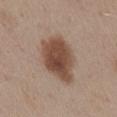This lesion was catalogued during total-body skin photography and was not selected for biopsy. Automated tile analysis of the lesion measured a footprint of about 20 mm², a shape eccentricity near 0.65, and a symmetry-axis asymmetry near 0.2. Captured under white-light illumination. A female patient, roughly 45 years of age. From the mid back. Approximately 5.5 mm at its widest. A roughly 15 mm field-of-view crop from a total-body skin photograph.